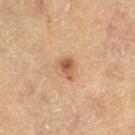Case summary:
* biopsy status: catalogued during a skin exam; not biopsied
* TBP lesion metrics: an automated nevus-likeness rating near 90 out of 100 and a lesion-detection confidence of about 100/100
* tile lighting: cross-polarized illumination
* image: ~15 mm crop, total-body skin-cancer survey
* location: the right thigh
* diameter: ~2.5 mm (longest diameter)
* subject: female, in their mid- to late 60s